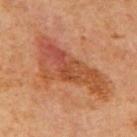Notes:
• notes — no biopsy performed (imaged during a skin exam)
• image-analysis metrics — an outline eccentricity of about 0.9 (0 = round, 1 = elongated); a mean CIELAB color near L≈43 a*≈24 b*≈32 and a lesion–skin lightness drop of about 9; a border-irregularity rating of about 6.5/10, a within-lesion color-variation index near 6.5/10, and a peripheral color-asymmetry measure near 2
• image — ~15 mm crop, total-body skin-cancer survey
• subject — male, about 65 years old
• tile lighting — cross-polarized illumination
• lesion diameter — ≈10.5 mm
• site — the mid back